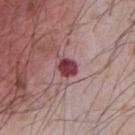workup: no biopsy performed (imaged during a skin exam) | lesion size: ~2.5 mm (longest diameter) | site: the front of the torso | automated metrics: a lesion area of about 4 mm² and two-axis asymmetry of about 0.2; about 18 CIELAB-L* units darker than the surrounding skin and a normalized lesion–skin contrast near 13; a border-irregularity rating of about 2/10, a color-variation rating of about 4.5/10, and radial color variation of about 1.5 | subject: male, aged 63 to 67 | tile lighting: white-light illumination | imaging modality: total-body-photography crop, ~15 mm field of view.